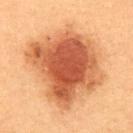| key | value |
|---|---|
| workup | catalogued during a skin exam; not biopsied |
| patient | male, aged 48 to 52 |
| site | the abdomen |
| tile lighting | cross-polarized illumination |
| acquisition | total-body-photography crop, ~15 mm field of view |
| lesion diameter | ~9 mm (longest diameter) |